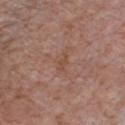lighting: white-light
patient: male, aged 58–62
automated metrics: a lesion area of about 2.5 mm², an outline eccentricity of about 0.9 (0 = round, 1 = elongated), and two-axis asymmetry of about 0.3; a mean CIELAB color near L≈48 a*≈20 b*≈28 and about 6 CIELAB-L* units darker than the surrounding skin; an automated nevus-likeness rating near 0 out of 100 and a detector confidence of about 100 out of 100 that the crop contains a lesion
lesion diameter: about 2.5 mm
acquisition: total-body-photography crop, ~15 mm field of view
site: the chest